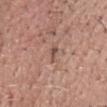{"biopsy_status": "not biopsied; imaged during a skin examination", "image": {"source": "total-body photography crop", "field_of_view_mm": 15}, "patient": {"sex": "male", "age_approx": 70}, "site": "head or neck"}